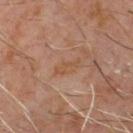workup — no biopsy performed (imaged during a skin exam) | patient — male, about 60 years old | location — the chest | acquisition — ~15 mm crop, total-body skin-cancer survey.